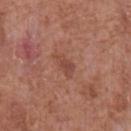Clinical impression: The lesion was photographed on a routine skin check and not biopsied; there is no pathology result. Background: A male patient, in their mid-60s. Cropped from a whole-body photographic skin survey; the tile spans about 15 mm. Located on the front of the torso. The tile uses white-light illumination.